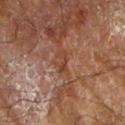Imaged during a routine full-body skin examination; the lesion was not biopsied and no histopathology is available. The tile uses cross-polarized illumination. A close-up tile cropped from a whole-body skin photograph, about 15 mm across. The lesion is located on the right forearm. The subject is a male in their mid-80s or older.